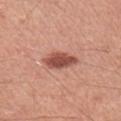| feature | finding |
|---|---|
| workup | catalogued during a skin exam; not biopsied |
| lesion diameter | ~4 mm (longest diameter) |
| patient | male, aged approximately 60 |
| body site | the right upper arm |
| lighting | white-light |
| imaging modality | 15 mm crop, total-body photography |
| automated lesion analysis | an eccentricity of roughly 0.8; an automated nevus-likeness rating near 95 out of 100 and a lesion-detection confidence of about 100/100 |